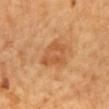| field | value |
|---|---|
| workup | no biopsy performed (imaged during a skin exam) |
| patient | male, aged 63 to 67 |
| body site | the mid back |
| tile lighting | cross-polarized illumination |
| acquisition | 15 mm crop, total-body photography |
| lesion diameter | ≈3.5 mm |